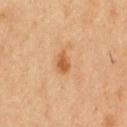Q: Was this lesion biopsied?
A: total-body-photography surveillance lesion; no biopsy
Q: Patient demographics?
A: male, aged approximately 50
Q: Where on the body is the lesion?
A: the chest
Q: How was this image acquired?
A: ~15 mm tile from a whole-body skin photo